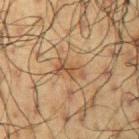Impression: Imaged during a routine full-body skin examination; the lesion was not biopsied and no histopathology is available. Acquisition and patient details: The tile uses cross-polarized illumination. A lesion tile, about 15 mm wide, cut from a 3D total-body photograph. About 3.5 mm across. A male subject, about 60 years old. Located on the arm.